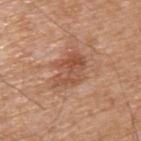Part of a total-body skin-imaging series; this lesion was reviewed on a skin check and was not flagged for biopsy.
Located on the upper back.
A male subject aged 63 to 67.
A region of skin cropped from a whole-body photographic capture, roughly 15 mm wide.
The tile uses white-light illumination.